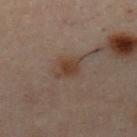No biopsy was performed on this lesion — it was imaged during a full skin examination and was not determined to be concerning.
The lesion is located on the chest.
The tile uses cross-polarized illumination.
Automated tile analysis of the lesion measured a mean CIELAB color near L≈31 a*≈13 b*≈21, roughly 6 lightness units darker than nearby skin, and a lesion-to-skin contrast of about 7.5 (normalized; higher = more distinct).
A male subject, approximately 30 years of age.
A close-up tile cropped from a whole-body skin photograph, about 15 mm across.
About 3 mm across.